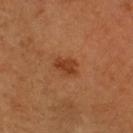Impression: The lesion was photographed on a routine skin check and not biopsied; there is no pathology result. Image and clinical context: Approximately 2.5 mm at its widest. The lesion is on the head or neck. The subject is a male aged 48 to 52. A roughly 15 mm field-of-view crop from a total-body skin photograph. The lesion-visualizer software estimated an area of roughly 4 mm² and a shape eccentricity near 0.65. It also reported a border-irregularity index near 3/10 and peripheral color asymmetry of about 0.5. The software also gave a classifier nevus-likeness of about 95/100. Captured under cross-polarized illumination.